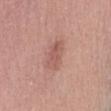- follow-up · imaged on a skin check; not biopsied
- body site · the lower back
- subject · female, aged around 40
- lighting · white-light
- acquisition · 15 mm crop, total-body photography